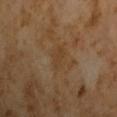Captured during whole-body skin photography for melanoma surveillance; the lesion was not biopsied.
The patient is a male aged 63–67.
A close-up tile cropped from a whole-body skin photograph, about 15 mm across.
The lesion-visualizer software estimated a nevus-likeness score of about 0/100 and a detector confidence of about 100 out of 100 that the crop contains a lesion.
The tile uses cross-polarized illumination.